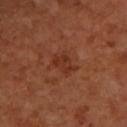tile lighting: cross-polarized illumination | size: about 3 mm | imaging modality: ~15 mm crop, total-body skin-cancer survey | anatomic site: the left forearm | automated metrics: an outline eccentricity of about 0.7 (0 = round, 1 = elongated) and a symmetry-axis asymmetry near 0.35; a lesion–skin lightness drop of about 7 and a normalized border contrast of about 6.5 | subject: female, aged approximately 50.